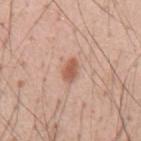Notes:
– workup · imaged on a skin check; not biopsied
– anatomic site · the back
– illumination · white-light
– subject · male, in their mid- to late 50s
– acquisition · ~15 mm crop, total-body skin-cancer survey
– size · ~2.5 mm (longest diameter)